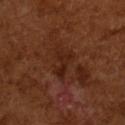Captured during whole-body skin photography for melanoma surveillance; the lesion was not biopsied.
Imaged with cross-polarized lighting.
A 15 mm close-up tile from a total-body photography series done for melanoma screening.
A male subject aged around 65.